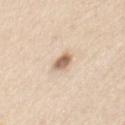Clinical impression:
This lesion was catalogued during total-body skin photography and was not selected for biopsy.
Image and clinical context:
The subject is a male aged approximately 40. Captured under white-light illumination. This image is a 15 mm lesion crop taken from a total-body photograph. Approximately 3 mm at its widest. On the chest.